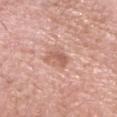Acquisition and patient details:
The patient is a male aged approximately 60. Measured at roughly 3 mm in maximum diameter. Cropped from a whole-body photographic skin survey; the tile spans about 15 mm. On the head or neck. This is a white-light tile.